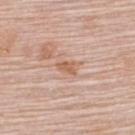<record>
  <patient>
    <sex>female</sex>
    <age_approx>65</age_approx>
  </patient>
  <image>
    <source>total-body photography crop</source>
    <field_of_view_mm>15</field_of_view_mm>
  </image>
  <site>upper back</site>
  <lesion_size>
    <long_diameter_mm_approx>2.5</long_diameter_mm_approx>
  </lesion_size>
  <lighting>white-light</lighting>
</record>